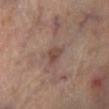Impression: The lesion was tiled from a total-body skin photograph and was not biopsied. Clinical summary: Cropped from a total-body skin-imaging series; the visible field is about 15 mm. Automated image analysis of the tile measured a border-irregularity index near 3/10, internal color variation of about 3 on a 0–10 scale, and radial color variation of about 1. The software also gave an automated nevus-likeness rating near 5 out of 100 and a detector confidence of about 100 out of 100 that the crop contains a lesion. A male patient, in their 70s. About 2.5 mm across. The lesion is on the left lower leg. This is a cross-polarized tile.